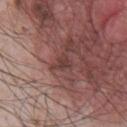follow-up: imaged on a skin check; not biopsied | body site: the chest | image: 15 mm crop, total-body photography | patient: male, aged approximately 65.